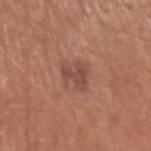Clinical impression: Part of a total-body skin-imaging series; this lesion was reviewed on a skin check and was not flagged for biopsy. Context: From the arm. An algorithmic analysis of the crop reported an area of roughly 5 mm², a shape eccentricity near 0.55, and a shape-asymmetry score of about 0.55 (0 = symmetric). It also reported a lesion color around L≈47 a*≈23 b*≈26 in CIELAB, roughly 8 lightness units darker than nearby skin, and a normalized border contrast of about 6.5. It also reported border irregularity of about 6.5 on a 0–10 scale, internal color variation of about 2 on a 0–10 scale, and radial color variation of about 0.5. A 15 mm close-up extracted from a 3D total-body photography capture. This is a white-light tile. A male subject, in their mid- to late 60s.